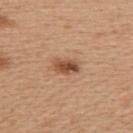Case summary:
- follow-up: imaged on a skin check; not biopsied
- location: the upper back
- subject: female, in their 40s
- lighting: white-light
- size: ~3 mm (longest diameter)
- image source: total-body-photography crop, ~15 mm field of view